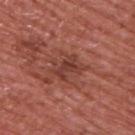Context: The lesion's longest dimension is about 4 mm. Automated image analysis of the tile measured lesion-presence confidence of about 100/100. A 15 mm close-up extracted from a 3D total-body photography capture. Located on the upper back. Captured under white-light illumination. The patient is a male aged approximately 65.